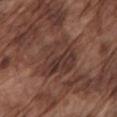The lesion is located on the arm.
This is a white-light tile.
Cropped from a total-body skin-imaging series; the visible field is about 15 mm.
The subject is a male aged 73 to 77.
The lesion-visualizer software estimated a lesion color around L≈36 a*≈19 b*≈22 in CIELAB, a lesion–skin lightness drop of about 7, and a normalized border contrast of about 6.5. The software also gave internal color variation of about 5.5 on a 0–10 scale and a peripheral color-asymmetry measure near 2.
The lesion's longest dimension is about 6 mm.